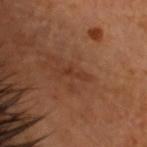| field | value |
|---|---|
| follow-up | imaged on a skin check; not biopsied |
| imaging modality | ~15 mm crop, total-body skin-cancer survey |
| image-analysis metrics | two-axis asymmetry of about 0.4; internal color variation of about 0 on a 0–10 scale |
| anatomic site | the head or neck |
| illumination | cross-polarized |
| patient | male, aged 48 to 52 |
| diameter | about 3 mm |